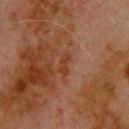{
  "biopsy_status": "not biopsied; imaged during a skin examination",
  "patient": {
    "sex": "male",
    "age_approx": 80
  },
  "image": {
    "source": "total-body photography crop",
    "field_of_view_mm": 15
  },
  "lesion_size": {
    "long_diameter_mm_approx": 3.0
  },
  "site": "back",
  "automated_metrics": {
    "vs_skin_darker_L": 6.0,
    "vs_skin_contrast_norm": 7.5
  }
}